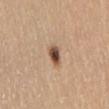Imaged during a routine full-body skin examination; the lesion was not biopsied and no histopathology is available.
Cropped from a whole-body photographic skin survey; the tile spans about 15 mm.
The lesion is located on the abdomen.
The tile uses white-light illumination.
Approximately 3 mm at its widest.
The total-body-photography lesion software estimated a border-irregularity rating of about 2.5/10, internal color variation of about 6.5 on a 0–10 scale, and radial color variation of about 2.
A female patient aged around 65.